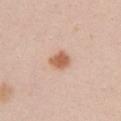imaging modality = 15 mm crop, total-body photography; subject = female, aged approximately 20; body site = the left upper arm.